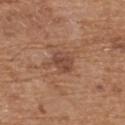Imaged during a routine full-body skin examination; the lesion was not biopsied and no histopathology is available.
A male subject, aged approximately 70.
The lesion is on the upper back.
Cropped from a whole-body photographic skin survey; the tile spans about 15 mm.